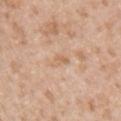This lesion was catalogued during total-body skin photography and was not selected for biopsy. The lesion's longest dimension is about 3 mm. Automated tile analysis of the lesion measured a footprint of about 2.5 mm² and an outline eccentricity of about 0.9 (0 = round, 1 = elongated). The software also gave an average lesion color of about L≈64 a*≈19 b*≈34 (CIELAB) and a lesion-to-skin contrast of about 5 (normalized; higher = more distinct). The analysis additionally found a classifier nevus-likeness of about 0/100 and a lesion-detection confidence of about 100/100. A roughly 15 mm field-of-view crop from a total-body skin photograph. The subject is a male aged 33 to 37. This is a white-light tile. On the left upper arm.